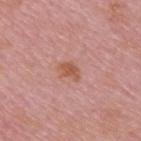<record>
<biopsy_status>not biopsied; imaged during a skin examination</biopsy_status>
<site>upper back</site>
<lighting>white-light</lighting>
<image>
  <source>total-body photography crop</source>
  <field_of_view_mm>15</field_of_view_mm>
</image>
<lesion_size>
  <long_diameter_mm_approx>2.5</long_diameter_mm_approx>
</lesion_size>
<patient>
  <sex>male</sex>
  <age_approx>50</age_approx>
</patient>
</record>